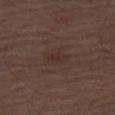Recorded during total-body skin imaging; not selected for excision or biopsy. A male subject, aged 68 to 72. The tile uses white-light illumination. A roughly 15 mm field-of-view crop from a total-body skin photograph. About 2.5 mm across. On the right thigh.